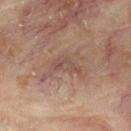A 15 mm crop from a total-body photograph taken for skin-cancer surveillance. A male subject aged 83 or over. About 3.5 mm across. An algorithmic analysis of the crop reported a mean CIELAB color near L≈47 a*≈18 b*≈23, a lesion–skin lightness drop of about 7, and a normalized border contrast of about 5.5. The analysis additionally found a nevus-likeness score of about 0/100 and lesion-presence confidence of about 55/100. This is a cross-polarized tile. On the upper back.